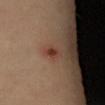Case summary:
• workup · imaged on a skin check; not biopsied
• patient · female, roughly 65 years of age
• illumination · cross-polarized
• lesion diameter · ≈2 mm
• image source · 15 mm crop, total-body photography
• anatomic site · the right forearm